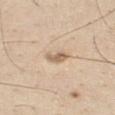This lesion was catalogued during total-body skin photography and was not selected for biopsy. Approximately 2.5 mm at its widest. Automated image analysis of the tile measured a lesion area of about 3 mm², a shape eccentricity near 0.85, and two-axis asymmetry of about 0.35. The analysis additionally found an automated nevus-likeness rating near 10 out of 100 and lesion-presence confidence of about 100/100. A male subject, aged around 30. From the chest. Cropped from a total-body skin-imaging series; the visible field is about 15 mm.